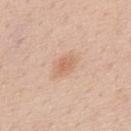Clinical impression:
The lesion was tiled from a total-body skin photograph and was not biopsied.
Clinical summary:
From the back. About 3 mm across. Cropped from a whole-body photographic skin survey; the tile spans about 15 mm. An algorithmic analysis of the crop reported an area of roughly 4.5 mm², an outline eccentricity of about 0.75 (0 = round, 1 = elongated), and a shape-asymmetry score of about 0.2 (0 = symmetric). And it measured a nevus-likeness score of about 40/100. A male subject, roughly 60 years of age.